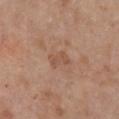{
  "biopsy_status": "not biopsied; imaged during a skin examination",
  "lesion_size": {
    "long_diameter_mm_approx": 3.0
  },
  "patient": {
    "sex": "female",
    "age_approx": 65
  },
  "automated_metrics": {
    "area_mm2_approx": 4.0,
    "eccentricity": 0.75,
    "shape_asymmetry": 0.25,
    "cielab_L": 52,
    "cielab_a": 20,
    "cielab_b": 30,
    "vs_skin_darker_L": 7.0,
    "vs_skin_contrast_norm": 5.0,
    "color_variation_0_10": 2.0,
    "peripheral_color_asymmetry": 1.0
  },
  "image": {
    "source": "total-body photography crop",
    "field_of_view_mm": 15
  },
  "site": "chest",
  "lighting": "white-light"
}